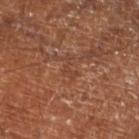The lesion is on the left lower leg.
The subject is aged 63 to 67.
This is a cross-polarized tile.
The total-body-photography lesion software estimated a lesion area of about 2.5 mm², an outline eccentricity of about 0.85 (0 = round, 1 = elongated), and two-axis asymmetry of about 0.7. The analysis additionally found a border-irregularity index near 7.5/10, internal color variation of about 0 on a 0–10 scale, and a peripheral color-asymmetry measure near 0. The software also gave an automated nevus-likeness rating near 0 out of 100 and lesion-presence confidence of about 60/100.
The recorded lesion diameter is about 2.5 mm.
A 15 mm close-up tile from a total-body photography series done for melanoma screening.